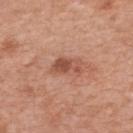The lesion was photographed on a routine skin check and not biopsied; there is no pathology result. A female subject, aged 33–37. This image is a 15 mm lesion crop taken from a total-body photograph. Located on the back.